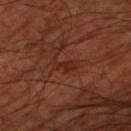{"biopsy_status": "not biopsied; imaged during a skin examination", "site": "left thigh", "lesion_size": {"long_diameter_mm_approx": 3.0}, "lighting": "cross-polarized", "image": {"source": "total-body photography crop", "field_of_view_mm": 15}, "automated_metrics": {"nevus_likeness_0_100": 0, "lesion_detection_confidence_0_100": 95}, "patient": {"age_approx": 65}}